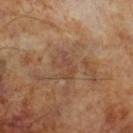Impression:
Imaged during a routine full-body skin examination; the lesion was not biopsied and no histopathology is available.
Acquisition and patient details:
A region of skin cropped from a whole-body photographic capture, roughly 15 mm wide. A male patient, aged 63 to 67. Measured at roughly 3.5 mm in maximum diameter. Captured under cross-polarized illumination.